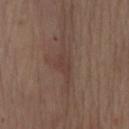The lesion was tiled from a total-body skin photograph and was not biopsied.
A roughly 15 mm field-of-view crop from a total-body skin photograph.
The lesion's longest dimension is about 4.5 mm.
The patient is a male in their 70s.
On the mid back.
Imaged with white-light lighting.